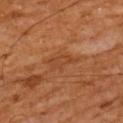<lesion>
  <patient>
    <sex>male</sex>
    <age_approx>65</age_approx>
  </patient>
  <image>
    <source>total-body photography crop</source>
    <field_of_view_mm>15</field_of_view_mm>
  </image>
  <lighting>cross-polarized</lighting>
  <site>upper back</site>
  <lesion_size>
    <long_diameter_mm_approx>3.5</long_diameter_mm_approx>
  </lesion_size>
  <automated_metrics>
    <area_mm2_approx>5.0</area_mm2_approx>
    <shape_asymmetry>0.35</shape_asymmetry>
    <lesion_detection_confidence_0_100>95</lesion_detection_confidence_0_100>
  </automated_metrics>
</lesion>